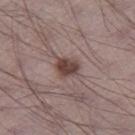Part of a total-body skin-imaging series; this lesion was reviewed on a skin check and was not flagged for biopsy. The lesion is on the leg. A 15 mm crop from a total-body photograph taken for skin-cancer surveillance. A male patient, aged approximately 70. Approximately 3 mm at its widest.